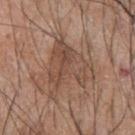Clinical impression: Imaged during a routine full-body skin examination; the lesion was not biopsied and no histopathology is available. Acquisition and patient details: The lesion-visualizer software estimated an eccentricity of roughly 0.3 and a shape-asymmetry score of about 0.55 (0 = symmetric). It also reported a mean CIELAB color near L≈48 a*≈18 b*≈27 and a lesion-to-skin contrast of about 6 (normalized; higher = more distinct). It also reported a border-irregularity rating of about 7/10, a color-variation rating of about 5/10, and peripheral color asymmetry of about 2. The software also gave a nevus-likeness score of about 0/100 and a detector confidence of about 95 out of 100 that the crop contains a lesion. The recorded lesion diameter is about 7 mm. This image is a 15 mm lesion crop taken from a total-body photograph. The lesion is located on the upper back. The subject is a male roughly 45 years of age.